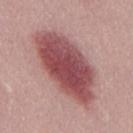This lesion was catalogued during total-body skin photography and was not selected for biopsy. A 15 mm close-up tile from a total-body photography series done for melanoma screening. The subject is a male approximately 30 years of age. The tile uses white-light illumination.